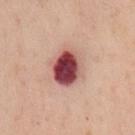Imaged during a routine full-body skin examination; the lesion was not biopsied and no histopathology is available. A lesion tile, about 15 mm wide, cut from a 3D total-body photograph. From the back. The tile uses cross-polarized illumination. The patient is a male in their mid- to late 50s. Longest diameter approximately 4.5 mm.